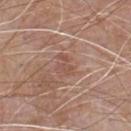Context:
The recorded lesion diameter is about 3 mm. Imaged with white-light lighting. Cropped from a whole-body photographic skin survey; the tile spans about 15 mm. The lesion is located on the front of the torso. A male subject approximately 65 years of age. An algorithmic analysis of the crop reported an area of roughly 4 mm² and two-axis asymmetry of about 0.45. And it measured an average lesion color of about L≈52 a*≈21 b*≈26 (CIELAB) and a lesion–skin lightness drop of about 6. The software also gave a border-irregularity rating of about 5.5/10, internal color variation of about 1 on a 0–10 scale, and peripheral color asymmetry of about 0.5. And it measured an automated nevus-likeness rating near 0 out of 100.